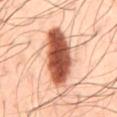imaging modality — 15 mm crop, total-body photography; size — about 8.5 mm; site — the mid back; patient — male, aged around 50.